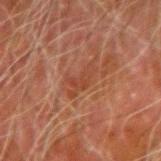No biopsy was performed on this lesion — it was imaged during a full skin examination and was not determined to be concerning. A 15 mm close-up tile from a total-body photography series done for melanoma screening. The lesion is on the arm. The subject is a male approximately 80 years of age.